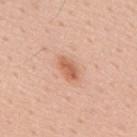Clinical impression: No biopsy was performed on this lesion — it was imaged during a full skin examination and was not determined to be concerning. Clinical summary: This is a white-light tile. The patient is a male in their mid-50s. A close-up tile cropped from a whole-body skin photograph, about 15 mm across. The lesion's longest dimension is about 3 mm. From the back.